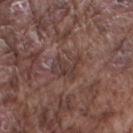Findings:
- workup — catalogued during a skin exam; not biopsied
- acquisition — 15 mm crop, total-body photography
- subject — male, about 75 years old
- lighting — white-light illumination
- anatomic site — the right upper arm